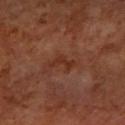| key | value |
|---|---|
| follow-up | total-body-photography surveillance lesion; no biopsy |
| patient | female, about 65 years old |
| acquisition | ~15 mm tile from a whole-body skin photo |
| diameter | ≈3 mm |
| illumination | cross-polarized |
| site | the left forearm |
| automated metrics | a lesion area of about 3.5 mm², an outline eccentricity of about 0.9 (0 = round, 1 = elongated), and a symmetry-axis asymmetry near 0.6; an automated nevus-likeness rating near 0 out of 100 and lesion-presence confidence of about 100/100 |